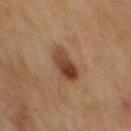  biopsy_status: not biopsied; imaged during a skin examination
  image:
    source: total-body photography crop
    field_of_view_mm: 15
  site: mid back
  patient:
    sex: male
    age_approx: 70